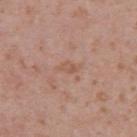{"biopsy_status": "not biopsied; imaged during a skin examination"}